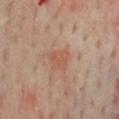Assessment:
This lesion was catalogued during total-body skin photography and was not selected for biopsy.
Background:
Imaged with cross-polarized lighting. A male patient aged 63–67. The lesion's longest dimension is about 2.5 mm. Automated image analysis of the tile measured a mean CIELAB color near L≈51 a*≈21 b*≈28 and about 6 CIELAB-L* units darker than the surrounding skin. A region of skin cropped from a whole-body photographic capture, roughly 15 mm wide. The lesion is located on the chest.